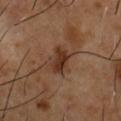Q: Is there a histopathology result?
A: total-body-photography surveillance lesion; no biopsy
Q: What kind of image is this?
A: ~15 mm crop, total-body skin-cancer survey
Q: How large is the lesion?
A: ~3.5 mm (longest diameter)
Q: What did automated image analysis measure?
A: a mean CIELAB color near L≈32 a*≈20 b*≈27, about 10 CIELAB-L* units darker than the surrounding skin, and a normalized border contrast of about 10
Q: What is the anatomic site?
A: the chest
Q: What are the patient's age and sex?
A: male, in their mid-50s
Q: How was the tile lit?
A: cross-polarized